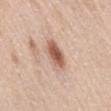{"biopsy_status": "not biopsied; imaged during a skin examination", "image": {"source": "total-body photography crop", "field_of_view_mm": 15}, "lesion_size": {"long_diameter_mm_approx": 4.0}, "lighting": "white-light", "automated_metrics": {"area_mm2_approx": 8.5, "eccentricity": 0.8, "shape_asymmetry": 0.2, "nevus_likeness_0_100": 95, "lesion_detection_confidence_0_100": 100}, "patient": {"sex": "female", "age_approx": 65}, "site": "right thigh"}